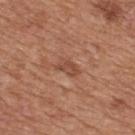Part of a total-body skin-imaging series; this lesion was reviewed on a skin check and was not flagged for biopsy. This image is a 15 mm lesion crop taken from a total-body photograph. The patient is a male about 65 years old. On the upper back. About 3 mm across.